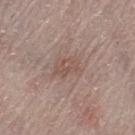The lesion was photographed on a routine skin check and not biopsied; there is no pathology result. A lesion tile, about 15 mm wide, cut from a 3D total-body photograph. The total-body-photography lesion software estimated a lesion–skin lightness drop of about 6 and a normalized lesion–skin contrast near 5. The software also gave a classifier nevus-likeness of about 0/100 and a detector confidence of about 100 out of 100 that the crop contains a lesion. The lesion is located on the left thigh. The recorded lesion diameter is about 3.5 mm. Imaged with white-light lighting. The patient is a female aged around 65.